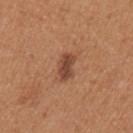  image:
    source: total-body photography crop
    field_of_view_mm: 15
  automated_metrics:
    border_irregularity_0_10: 2.5
    color_variation_0_10: 2.5
  site: left upper arm
  lesion_size:
    long_diameter_mm_approx: 3.0
  lighting: white-light
  patient:
    sex: male
    age_approx: 30